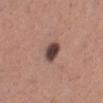Clinical impression:
The lesion was photographed on a routine skin check and not biopsied; there is no pathology result.
Context:
Imaged with white-light lighting. Longest diameter approximately 2.5 mm. Automated tile analysis of the lesion measured a lesion color around L≈42 a*≈18 b*≈20 in CIELAB and about 18 CIELAB-L* units darker than the surrounding skin. The analysis additionally found border irregularity of about 2 on a 0–10 scale, internal color variation of about 3.5 on a 0–10 scale, and radial color variation of about 1. The analysis additionally found an automated nevus-likeness rating near 35 out of 100 and a lesion-detection confidence of about 100/100. The patient is a female approximately 30 years of age. A region of skin cropped from a whole-body photographic capture, roughly 15 mm wide. Located on the leg.